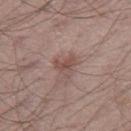<record>
<biopsy_status>not biopsied; imaged during a skin examination</biopsy_status>
<patient>
  <sex>male</sex>
  <age_approx>50</age_approx>
</patient>
<lesion_size>
  <long_diameter_mm_approx>3.0</long_diameter_mm_approx>
</lesion_size>
<lighting>white-light</lighting>
<image>
  <source>total-body photography crop</source>
  <field_of_view_mm>15</field_of_view_mm>
</image>
<site>leg</site>
</record>